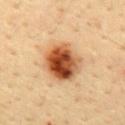Case summary:
• notes · catalogued during a skin exam; not biopsied
• diameter · about 5.5 mm
• automated metrics · a lesion color around L≈53 a*≈26 b*≈39 in CIELAB and a lesion–skin lightness drop of about 20; a border-irregularity rating of about 1.5/10, a within-lesion color-variation index near 10/10, and a peripheral color-asymmetry measure near 4.5
• image · total-body-photography crop, ~15 mm field of view
• patient · male, aged approximately 45
• illumination · cross-polarized
• site · the mid back